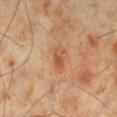Clinical impression: Captured during whole-body skin photography for melanoma surveillance; the lesion was not biopsied. Clinical summary: Cropped from a whole-body photographic skin survey; the tile spans about 15 mm. Longest diameter approximately 2.5 mm. Captured under cross-polarized illumination. The lesion is on the left lower leg. A male subject roughly 70 years of age.